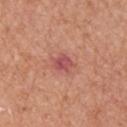Assessment: Imaged during a routine full-body skin examination; the lesion was not biopsied and no histopathology is available. Image and clinical context: Cropped from a total-body skin-imaging series; the visible field is about 15 mm. A male subject, in their mid- to late 60s. The recorded lesion diameter is about 3 mm. From the right upper arm.